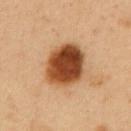  site: abdomen
  lesion_size:
    long_diameter_mm_approx: 5.5
  image:
    source: total-body photography crop
    field_of_view_mm: 15
  automated_metrics:
    area_mm2_approx: 20.0
    eccentricity: 0.65
    border_irregularity_0_10: 1.5
    color_variation_0_10: 7.5
    peripheral_color_asymmetry: 2.5
    nevus_likeness_0_100: 100
    lesion_detection_confidence_0_100: 100
  patient:
    sex: male
    age_approx: 50